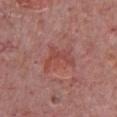Clinical summary:
Located on the chest. A close-up tile cropped from a whole-body skin photograph, about 15 mm across. A male patient aged 73 to 77.